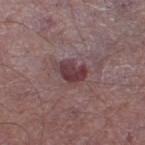Assessment:
The lesion was tiled from a total-body skin photograph and was not biopsied.
Clinical summary:
Approximately 3.5 mm at its widest. This image is a 15 mm lesion crop taken from a total-body photograph. Imaged with white-light lighting. An algorithmic analysis of the crop reported a footprint of about 6.5 mm², an eccentricity of roughly 0.7, and a shape-asymmetry score of about 0.3 (0 = symmetric). The lesion is located on the right lower leg. A male patient, aged 63 to 67.